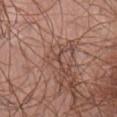biopsy status=catalogued during a skin exam; not biopsied.